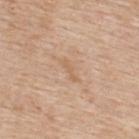Part of a total-body skin-imaging series; this lesion was reviewed on a skin check and was not flagged for biopsy.
Cropped from a total-body skin-imaging series; the visible field is about 15 mm.
The lesion is on the upper back.
A male patient, aged 58–62.